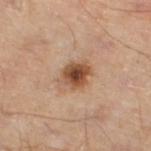Q: Is there a histopathology result?
A: catalogued during a skin exam; not biopsied
Q: What is the imaging modality?
A: total-body-photography crop, ~15 mm field of view
Q: Patient demographics?
A: male, aged 43 to 47
Q: Where on the body is the lesion?
A: the left lower leg